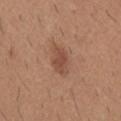| feature | finding |
|---|---|
| acquisition | ~15 mm tile from a whole-body skin photo |
| patient | male, aged 58 to 62 |
| automated lesion analysis | a lesion color around L≈49 a*≈21 b*≈29 in CIELAB and a lesion-to-skin contrast of about 6.5 (normalized; higher = more distinct); a color-variation rating of about 2/10 and radial color variation of about 0.5; a classifier nevus-likeness of about 80/100 and lesion-presence confidence of about 100/100 |
| location | the mid back |